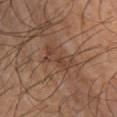Case summary:
– follow-up: total-body-photography surveillance lesion; no biopsy
– patient: male, roughly 65 years of age
– size: ≈4.5 mm
– site: the right lower leg
– image: ~15 mm crop, total-body skin-cancer survey
– illumination: cross-polarized